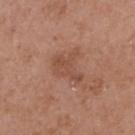Findings:
• notes · total-body-photography surveillance lesion; no biopsy
• subject · female, aged 38–42
• lighting · white-light illumination
• image source · total-body-photography crop, ~15 mm field of view
• site · the upper back
• size · ≈3.5 mm